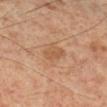The lesion was photographed on a routine skin check and not biopsied; there is no pathology result. Located on the right lower leg. Automated image analysis of the tile measured an automated nevus-likeness rating near 0 out of 100 and a detector confidence of about 100 out of 100 that the crop contains a lesion. Cropped from a total-body skin-imaging series; the visible field is about 15 mm. The tile uses cross-polarized illumination. The subject is a male about 55 years old.